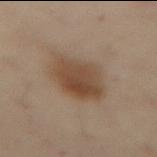Impression:
The lesion was photographed on a routine skin check and not biopsied; there is no pathology result.
Clinical summary:
The lesion is on the abdomen. About 5.5 mm across. A close-up tile cropped from a whole-body skin photograph, about 15 mm across. The tile uses cross-polarized illumination. A female subject, roughly 55 years of age.